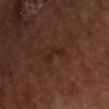The lesion was tiled from a total-body skin photograph and was not biopsied. A female subject aged approximately 40. A roughly 15 mm field-of-view crop from a total-body skin photograph. Located on the chest.